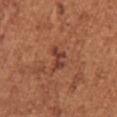  biopsy_status: not biopsied; imaged during a skin examination
  site: right upper arm
  lesion_size:
    long_diameter_mm_approx: 3.0
  lighting: white-light
  patient:
    sex: female
    age_approx: 50
  image:
    source: total-body photography crop
    field_of_view_mm: 15